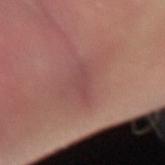The lesion-visualizer software estimated an average lesion color of about L≈47 a*≈25 b*≈21 (CIELAB), about 6 CIELAB-L* units darker than the surrounding skin, and a normalized border contrast of about 5. The analysis additionally found a border-irregularity rating of about 3.5/10, internal color variation of about 1.5 on a 0–10 scale, and radial color variation of about 0.5.
Cropped from a whole-body photographic skin survey; the tile spans about 15 mm.
Imaged with white-light lighting.
From the right forearm.
About 3.5 mm across.
A male subject aged around 35.